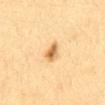Impression: The lesion was tiled from a total-body skin photograph and was not biopsied. Clinical summary: Located on the abdomen. A female subject in their 30s. A close-up tile cropped from a whole-body skin photograph, about 15 mm across. The tile uses cross-polarized illumination. Automated image analysis of the tile measured a lesion-detection confidence of about 100/100. The recorded lesion diameter is about 2.5 mm.